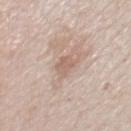Part of a total-body skin-imaging series; this lesion was reviewed on a skin check and was not flagged for biopsy.
Captured under white-light illumination.
The lesion's longest dimension is about 2.5 mm.
Located on the chest.
An algorithmic analysis of the crop reported a lesion area of about 4 mm², an outline eccentricity of about 0.7 (0 = round, 1 = elongated), and a shape-asymmetry score of about 0.25 (0 = symmetric). And it measured an automated nevus-likeness rating near 0 out of 100 and lesion-presence confidence of about 95/100.
A male subject about 60 years old.
A region of skin cropped from a whole-body photographic capture, roughly 15 mm wide.